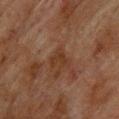biopsy status: imaged on a skin check; not biopsied | tile lighting: cross-polarized | anatomic site: the upper back | image-analysis metrics: an area of roughly 4.5 mm², an eccentricity of roughly 0.55, and a shape-asymmetry score of about 0.45 (0 = symmetric); a mean CIELAB color near L≈29 a*≈17 b*≈25, a lesion–skin lightness drop of about 5, and a lesion-to-skin contrast of about 6 (normalized; higher = more distinct) | lesion size: about 3 mm | subject: male, in their mid-70s | image: total-body-photography crop, ~15 mm field of view.